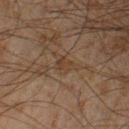* workup · imaged on a skin check; not biopsied
* patient · male, roughly 45 years of age
* lighting · cross-polarized illumination
* automated lesion analysis · an average lesion color of about L≈31 a*≈13 b*≈23 (CIELAB), about 4 CIELAB-L* units darker than the surrounding skin, and a lesion-to-skin contrast of about 5 (normalized; higher = more distinct); border irregularity of about 5 on a 0–10 scale, a color-variation rating of about 2.5/10, and peripheral color asymmetry of about 1; a nevus-likeness score of about 0/100 and lesion-presence confidence of about 70/100
* site · the right lower leg
* acquisition · ~15 mm tile from a whole-body skin photo
* lesion size · ≈3 mm